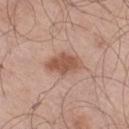{
  "biopsy_status": "not biopsied; imaged during a skin examination",
  "lighting": "white-light",
  "automated_metrics": {
    "border_irregularity_0_10": 2.5,
    "color_variation_0_10": 2.0,
    "peripheral_color_asymmetry": 0.5
  },
  "patient": {
    "sex": "male",
    "age_approx": 70
  },
  "site": "right thigh",
  "image": {
    "source": "total-body photography crop",
    "field_of_view_mm": 15
  },
  "lesion_size": {
    "long_diameter_mm_approx": 4.0
  }
}